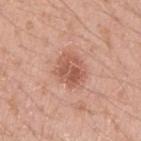Clinical impression: Part of a total-body skin-imaging series; this lesion was reviewed on a skin check and was not flagged for biopsy. Clinical summary: The lesion-visualizer software estimated a lesion–skin lightness drop of about 11 and a normalized lesion–skin contrast near 7. About 4 mm across. This is a white-light tile. A male patient roughly 20 years of age. A lesion tile, about 15 mm wide, cut from a 3D total-body photograph. From the left upper arm.